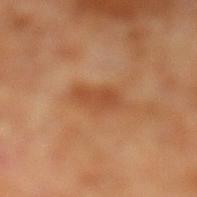A male subject, aged 58 to 62.
From the leg.
A roughly 15 mm field-of-view crop from a total-body skin photograph.
This is a cross-polarized tile.
The recorded lesion diameter is about 4 mm.